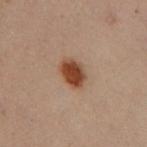Q: Was a biopsy performed?
A: imaged on a skin check; not biopsied
Q: Lesion location?
A: the left arm
Q: What kind of image is this?
A: 15 mm crop, total-body photography
Q: Lesion size?
A: ≈3.5 mm
Q: Patient demographics?
A: female, aged around 30
Q: Illumination type?
A: cross-polarized illumination
Q: What did automated image analysis measure?
A: a shape eccentricity near 0.6 and a symmetry-axis asymmetry near 0.2; border irregularity of about 1.5 on a 0–10 scale, a color-variation rating of about 3.5/10, and radial color variation of about 1